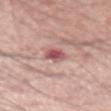Q: Is there a histopathology result?
A: catalogued during a skin exam; not biopsied
Q: What did automated image analysis measure?
A: an area of roughly 4.5 mm²; border irregularity of about 2 on a 0–10 scale, a color-variation rating of about 5/10, and radial color variation of about 1.5; a classifier nevus-likeness of about 5/100 and lesion-presence confidence of about 100/100
Q: What is the lesion's diameter?
A: ≈3 mm
Q: Where on the body is the lesion?
A: the arm
Q: What is the imaging modality?
A: total-body-photography crop, ~15 mm field of view
Q: Patient demographics?
A: male, aged approximately 60